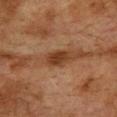biopsy status=total-body-photography surveillance lesion; no biopsy
lesion size=about 3 mm
patient=male, aged around 75
acquisition=total-body-photography crop, ~15 mm field of view
body site=the upper back